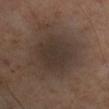notes: imaged on a skin check; not biopsied | automated lesion analysis: a footprint of about 21 mm², an outline eccentricity of about 0.5 (0 = round, 1 = elongated), and a symmetry-axis asymmetry near 0.15; border irregularity of about 1.5 on a 0–10 scale, internal color variation of about 3 on a 0–10 scale, and a peripheral color-asymmetry measure near 1 | diameter: about 5.5 mm | imaging modality: ~15 mm crop, total-body skin-cancer survey | subject: male, in their mid-50s | body site: the right upper arm.